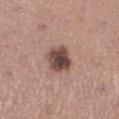Impression: The lesion was photographed on a routine skin check and not biopsied; there is no pathology result. Clinical summary: This is a white-light tile. The lesion is located on the leg. The lesion's longest dimension is about 3.5 mm. The total-body-photography lesion software estimated an area of roughly 9.5 mm², an eccentricity of roughly 0.3, and a symmetry-axis asymmetry near 0.15. It also reported a lesion color around L≈46 a*≈18 b*≈22 in CIELAB, roughly 16 lightness units darker than nearby skin, and a normalized lesion–skin contrast near 11.5. The software also gave border irregularity of about 1.5 on a 0–10 scale and radial color variation of about 1.5. And it measured a nevus-likeness score of about 35/100 and a detector confidence of about 100 out of 100 that the crop contains a lesion. A female subject, approximately 30 years of age. A 15 mm crop from a total-body photograph taken for skin-cancer surveillance.